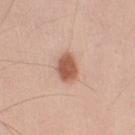Assessment: The lesion was tiled from a total-body skin photograph and was not biopsied. Background: The lesion is on the right upper arm. Captured under white-light illumination. A male subject, approximately 35 years of age. About 3.5 mm across. A 15 mm close-up tile from a total-body photography series done for melanoma screening.